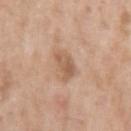Findings:
- workup: total-body-photography surveillance lesion; no biopsy
- body site: the left upper arm
- subject: male, aged 48 to 52
- imaging modality: ~15 mm crop, total-body skin-cancer survey
- lesion size: ~3 mm (longest diameter)